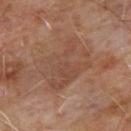Impression:
Imaged during a routine full-body skin examination; the lesion was not biopsied and no histopathology is available.
Context:
From the upper back. A 15 mm crop from a total-body photograph taken for skin-cancer surveillance. A male patient, approximately 65 years of age.